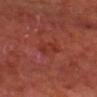Captured during whole-body skin photography for melanoma surveillance; the lesion was not biopsied. The total-body-photography lesion software estimated a footprint of about 4.5 mm², a shape eccentricity near 0.8, and a symmetry-axis asymmetry near 0.35. It also reported an automated nevus-likeness rating near 0 out of 100. Located on the head or neck. A region of skin cropped from a whole-body photographic capture, roughly 15 mm wide. The tile uses cross-polarized illumination. A male subject roughly 65 years of age.